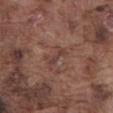Q: What are the patient's age and sex?
A: male, about 75 years old
Q: Where on the body is the lesion?
A: the front of the torso
Q: What kind of image is this?
A: ~15 mm tile from a whole-body skin photo